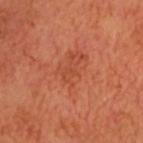Q: Was a biopsy performed?
A: no biopsy performed (imaged during a skin exam)
Q: What kind of image is this?
A: ~15 mm crop, total-body skin-cancer survey
Q: Where on the body is the lesion?
A: the head or neck
Q: Illumination type?
A: cross-polarized
Q: Who is the patient?
A: male, aged approximately 65